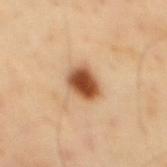workup — no biopsy performed (imaged during a skin exam)
size — about 3.5 mm
imaging modality — total-body-photography crop, ~15 mm field of view
location — the mid back
image-analysis metrics — an average lesion color of about L≈52 a*≈23 b*≈37 (CIELAB) and a lesion-to-skin contrast of about 12.5 (normalized; higher = more distinct); a border-irregularity index near 1.5/10, a color-variation rating of about 6/10, and a peripheral color-asymmetry measure near 1.5
patient — male, aged 38–42
illumination — cross-polarized